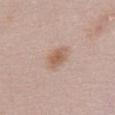follow-up: total-body-photography surveillance lesion; no biopsy
imaging modality: total-body-photography crop, ~15 mm field of view
patient: female, aged 48 to 52
automated lesion analysis: a lesion area of about 5 mm² and a shape-asymmetry score of about 0.3 (0 = symmetric); a classifier nevus-likeness of about 80/100 and a detector confidence of about 100 out of 100 that the crop contains a lesion
lesion diameter: ~3 mm (longest diameter)
tile lighting: white-light illumination
body site: the front of the torso